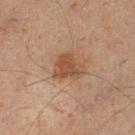Assessment: Recorded during total-body skin imaging; not selected for excision or biopsy. Background: From the left lower leg. A 15 mm crop from a total-body photograph taken for skin-cancer surveillance. The patient is a male aged approximately 50.